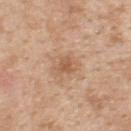Impression:
Recorded during total-body skin imaging; not selected for excision or biopsy.
Context:
Imaged with white-light lighting. A 15 mm crop from a total-body photograph taken for skin-cancer surveillance. Approximately 2.5 mm at its widest. A male subject aged 48 to 52. Located on the upper back.